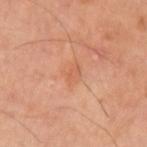biopsy status: no biopsy performed (imaged during a skin exam) | automated lesion analysis: a lesion area of about 4 mm², an outline eccentricity of about 0.8 (0 = round, 1 = elongated), and a symmetry-axis asymmetry near 0.3; a lesion color around L≈60 a*≈26 b*≈36 in CIELAB, about 6 CIELAB-L* units darker than the surrounding skin, and a normalized lesion–skin contrast near 4.5; a border-irregularity rating of about 3/10 and radial color variation of about 0.5 | acquisition: ~15 mm crop, total-body skin-cancer survey | lesion diameter: about 3 mm | anatomic site: the left upper arm | lighting: cross-polarized | patient: male, aged 38–42.